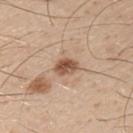Clinical impression:
This lesion was catalogued during total-body skin photography and was not selected for biopsy.
Clinical summary:
Approximately 3 mm at its widest. From the left upper arm. An algorithmic analysis of the crop reported a lesion area of about 4.5 mm², a shape eccentricity near 0.75, and a shape-asymmetry score of about 0.25 (0 = symmetric). It also reported a lesion color around L≈53 a*≈20 b*≈31 in CIELAB, roughly 14 lightness units darker than nearby skin, and a normalized lesion–skin contrast near 9.5. The software also gave an automated nevus-likeness rating near 65 out of 100 and a detector confidence of about 100 out of 100 that the crop contains a lesion. The subject is a male aged 28–32. A 15 mm crop from a total-body photograph taken for skin-cancer surveillance.